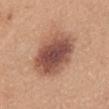This lesion was catalogued during total-body skin photography and was not selected for biopsy. The total-body-photography lesion software estimated a footprint of about 26 mm², an eccentricity of roughly 0.6, and a symmetry-axis asymmetry near 0.1. The software also gave a nevus-likeness score of about 70/100 and a lesion-detection confidence of about 100/100. From the back. A female patient, approximately 30 years of age. Cropped from a whole-body photographic skin survey; the tile spans about 15 mm. The tile uses white-light illumination.